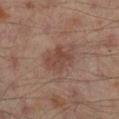The lesion was tiled from a total-body skin photograph and was not biopsied. The tile uses cross-polarized illumination. Located on the right lower leg. Automated tile analysis of the lesion measured a nevus-likeness score of about 5/100 and lesion-presence confidence of about 100/100. A 15 mm close-up tile from a total-body photography series done for melanoma screening. The recorded lesion diameter is about 3.5 mm. The patient is a male aged around 65.